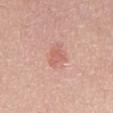Case summary:
• biopsy status: catalogued during a skin exam; not biopsied
• image: 15 mm crop, total-body photography
• lighting: white-light
• TBP lesion metrics: a border-irregularity rating of about 4.5/10 and peripheral color asymmetry of about 0.5; a classifier nevus-likeness of about 20/100 and a lesion-detection confidence of about 100/100
• lesion diameter: ≈3 mm
• anatomic site: the front of the torso
• patient: male, in their mid-40s